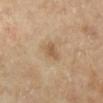Clinical summary: From the left lower leg. Cropped from a whole-body photographic skin survey; the tile spans about 15 mm. A female subject roughly 60 years of age. Imaged with cross-polarized lighting. Approximately 2.5 mm at its widest.